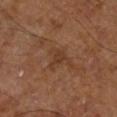Recorded during total-body skin imaging; not selected for excision or biopsy.
Automated tile analysis of the lesion measured a lesion area of about 4.5 mm² and two-axis asymmetry of about 0.65. The analysis additionally found a mean CIELAB color near L≈36 a*≈20 b*≈30, a lesion–skin lightness drop of about 6, and a normalized lesion–skin contrast near 5.5.
On the right lower leg.
The lesion's longest dimension is about 3 mm.
A lesion tile, about 15 mm wide, cut from a 3D total-body photograph.
A patient aged approximately 65.
Imaged with cross-polarized lighting.